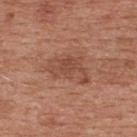Part of a total-body skin-imaging series; this lesion was reviewed on a skin check and was not flagged for biopsy.
A male patient approximately 30 years of age.
A roughly 15 mm field-of-view crop from a total-body skin photograph.
The tile uses white-light illumination.
The lesion is on the back.